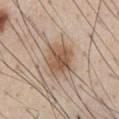Q: Was this lesion biopsied?
A: catalogued during a skin exam; not biopsied
Q: Illumination type?
A: white-light illumination
Q: What kind of image is this?
A: 15 mm crop, total-body photography
Q: Automated lesion metrics?
A: a mean CIELAB color near L≈55 a*≈16 b*≈30, roughly 11 lightness units darker than nearby skin, and a lesion-to-skin contrast of about 8 (normalized; higher = more distinct); border irregularity of about 2 on a 0–10 scale, a color-variation rating of about 4.5/10, and a peripheral color-asymmetry measure near 1.5; a nevus-likeness score of about 85/100 and a lesion-detection confidence of about 100/100
Q: What is the lesion's diameter?
A: about 4 mm
Q: What is the anatomic site?
A: the front of the torso
Q: What are the patient's age and sex?
A: male, approximately 60 years of age